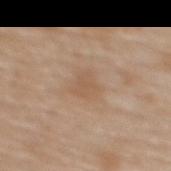Assessment: No biopsy was performed on this lesion — it was imaged during a full skin examination and was not determined to be concerning. Background: The lesion is located on the upper back. A roughly 15 mm field-of-view crop from a total-body skin photograph. A female subject, approximately 65 years of age.